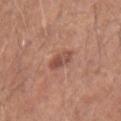Impression:
The lesion was tiled from a total-body skin photograph and was not biopsied.
Acquisition and patient details:
A male patient, in their 40s. A lesion tile, about 15 mm wide, cut from a 3D total-body photograph. Located on the right upper arm.